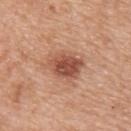<record>
<patient>
  <sex>female</sex>
  <age_approx>55</age_approx>
</patient>
<image>
  <source>total-body photography crop</source>
  <field_of_view_mm>15</field_of_view_mm>
</image>
<site>upper back</site>
</record>